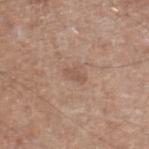Q: Is there a histopathology result?
A: no biopsy performed (imaged during a skin exam)
Q: What is the anatomic site?
A: the right lower leg
Q: How was this image acquired?
A: ~15 mm crop, total-body skin-cancer survey
Q: Illumination type?
A: white-light illumination
Q: What is the lesion's diameter?
A: ≈2.5 mm
Q: Patient demographics?
A: male, in their mid-60s
Q: What did automated image analysis measure?
A: an average lesion color of about L≈54 a*≈18 b*≈27 (CIELAB) and a normalized border contrast of about 5.5; a within-lesion color-variation index near 0.5/10; lesion-presence confidence of about 100/100